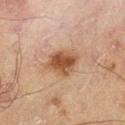Assessment: No biopsy was performed on this lesion — it was imaged during a full skin examination and was not determined to be concerning. Background: Longest diameter approximately 4 mm. A 15 mm close-up tile from a total-body photography series done for melanoma screening. From the left thigh. A male patient aged around 70. Imaged with cross-polarized lighting.